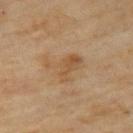{"biopsy_status": "not biopsied; imaged during a skin examination", "site": "left upper arm", "image": {"source": "total-body photography crop", "field_of_view_mm": 15}, "patient": {"sex": "female", "age_approx": 60}}